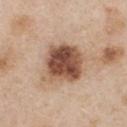workup: no biopsy performed (imaged during a skin exam) | automated lesion analysis: roughly 17 lightness units darker than nearby skin and a lesion-to-skin contrast of about 11.5 (normalized; higher = more distinct); border irregularity of about 2.5 on a 0–10 scale, a color-variation rating of about 7.5/10, and a peripheral color-asymmetry measure near 2 | body site: the chest | size: ~5.5 mm (longest diameter) | image source: 15 mm crop, total-body photography | illumination: white-light | subject: female, approximately 45 years of age.